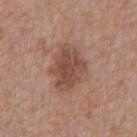Assessment:
This lesion was catalogued during total-body skin photography and was not selected for biopsy.
Image and clinical context:
A 15 mm close-up extracted from a 3D total-body photography capture. Measured at roughly 5 mm in maximum diameter. Imaged with white-light lighting. Automated image analysis of the tile measured a lesion–skin lightness drop of about 10 and a lesion-to-skin contrast of about 7.5 (normalized; higher = more distinct). The analysis additionally found a border-irregularity index near 2.5/10, internal color variation of about 4 on a 0–10 scale, and radial color variation of about 1.5. A male patient, aged 63 to 67. The lesion is on the front of the torso.